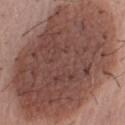• site — the right upper arm
• lighting — white-light illumination
• acquisition — ~15 mm crop, total-body skin-cancer survey
• lesion size — ≈15.5 mm
• TBP lesion metrics — a footprint of about 135 mm², an outline eccentricity of about 0.7 (0 = round, 1 = elongated), and two-axis asymmetry of about 0.1; about 14 CIELAB-L* units darker than the surrounding skin and a normalized border contrast of about 10; a within-lesion color-variation index near 5.5/10 and peripheral color asymmetry of about 2; a nevus-likeness score of about 0/100 and lesion-presence confidence of about 100/100
• subject — male, in their mid-70s